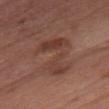This lesion was catalogued during total-body skin photography and was not selected for biopsy.
A female patient aged 58 to 62.
The lesion is located on the chest.
Cropped from a total-body skin-imaging series; the visible field is about 15 mm.